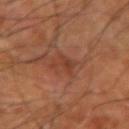Clinical impression:
Part of a total-body skin-imaging series; this lesion was reviewed on a skin check and was not flagged for biopsy.
Background:
This is a cross-polarized tile. The lesion is located on the arm. A 15 mm close-up tile from a total-body photography series done for melanoma screening. The patient is aged 63–67. Automated tile analysis of the lesion measured a footprint of about 4 mm² and two-axis asymmetry of about 0.5.